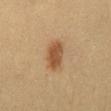workup = total-body-photography surveillance lesion; no biopsy
subject = female, aged approximately 30
body site = the abdomen
image = ~15 mm tile from a whole-body skin photo